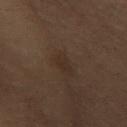Clinical impression:
Part of a total-body skin-imaging series; this lesion was reviewed on a skin check and was not flagged for biopsy.
Background:
Captured under cross-polarized illumination. The lesion is located on the chest. The subject is a female in their mid-50s. A lesion tile, about 15 mm wide, cut from a 3D total-body photograph. The total-body-photography lesion software estimated an area of roughly 3 mm², an eccentricity of roughly 0.85, and a shape-asymmetry score of about 0.2 (0 = symmetric). It also reported peripheral color asymmetry of about 0.5. It also reported a detector confidence of about 100 out of 100 that the crop contains a lesion.